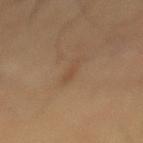Case summary:
- follow-up: catalogued during a skin exam; not biopsied
- subject: male, aged approximately 55
- image: ~15 mm crop, total-body skin-cancer survey
- site: the mid back